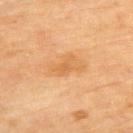Clinical impression:
The lesion was photographed on a routine skin check and not biopsied; there is no pathology result.